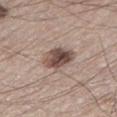Impression:
Captured during whole-body skin photography for melanoma surveillance; the lesion was not biopsied.
Acquisition and patient details:
Automated tile analysis of the lesion measured a border-irregularity rating of about 2/10, a color-variation rating of about 8/10, and a peripheral color-asymmetry measure near 3. It also reported a lesion-detection confidence of about 100/100. A male subject, aged 73–77. Imaged with white-light lighting. The lesion is on the left lower leg. A lesion tile, about 15 mm wide, cut from a 3D total-body photograph.